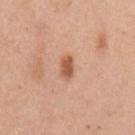– biopsy status: total-body-photography surveillance lesion; no biopsy
– anatomic site: the arm
– tile lighting: white-light
– subject: female, aged approximately 40
– acquisition: ~15 mm crop, total-body skin-cancer survey